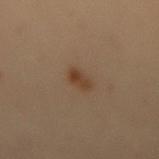biopsy status — imaged on a skin check; not biopsied | image — 15 mm crop, total-body photography | site — the mid back | subject — female, roughly 50 years of age.